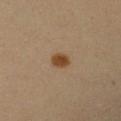| feature | finding |
|---|---|
| follow-up | no biopsy performed (imaged during a skin exam) |
| location | the left upper arm |
| subject | female, approximately 50 years of age |
| lighting | cross-polarized |
| TBP lesion metrics | a footprint of about 4 mm², an outline eccentricity of about 0.75 (0 = round, 1 = elongated), and two-axis asymmetry of about 0.15; an average lesion color of about L≈44 a*≈17 b*≈34 (CIELAB), a lesion–skin lightness drop of about 11, and a lesion-to-skin contrast of about 10 (normalized; higher = more distinct); a border-irregularity rating of about 1/10, a color-variation rating of about 2/10, and radial color variation of about 0.5; a nevus-likeness score of about 100/100 and lesion-presence confidence of about 100/100 |
| size | about 2.5 mm |
| image | ~15 mm tile from a whole-body skin photo |